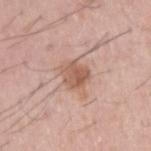{
  "biopsy_status": "not biopsied; imaged during a skin examination",
  "lighting": "white-light",
  "automated_metrics": {
    "shape_asymmetry": 0.2,
    "border_irregularity_0_10": 2.5,
    "color_variation_0_10": 3.5,
    "peripheral_color_asymmetry": 1.5
  },
  "image": {
    "source": "total-body photography crop",
    "field_of_view_mm": 15
  },
  "lesion_size": {
    "long_diameter_mm_approx": 3.0
  },
  "patient": {
    "sex": "male",
    "age_approx": 55
  }
}